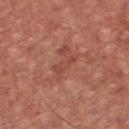Notes:
• workup — catalogued during a skin exam; not biopsied
• automated metrics — an area of roughly 3 mm², an eccentricity of roughly 0.9, and a symmetry-axis asymmetry near 0.35; a mean CIELAB color near L≈47 a*≈27 b*≈29, about 6 CIELAB-L* units darker than the surrounding skin, and a normalized lesion–skin contrast near 5; a border-irregularity rating of about 4/10 and a color-variation rating of about 0.5/10; an automated nevus-likeness rating near 0 out of 100 and a detector confidence of about 95 out of 100 that the crop contains a lesion
• imaging modality — total-body-photography crop, ~15 mm field of view
• location — the chest
• diameter — about 3 mm
• lighting — white-light illumination
• patient — male, about 65 years old